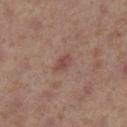Impression: The lesion was photographed on a routine skin check and not biopsied; there is no pathology result. Background: This image is a 15 mm lesion crop taken from a total-body photograph. Approximately 2.5 mm at its widest. This is a cross-polarized tile. The total-body-photography lesion software estimated an area of roughly 3.5 mm² and an eccentricity of roughly 0.8. It also reported an average lesion color of about L≈46 a*≈22 b*≈22 (CIELAB) and a lesion-to-skin contrast of about 6 (normalized; higher = more distinct). It also reported border irregularity of about 2 on a 0–10 scale and a color-variation rating of about 1.5/10. The lesion is on the left lower leg. A female patient, aged approximately 40.